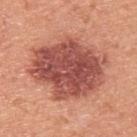Background: Automated tile analysis of the lesion measured a footprint of about 44 mm², an eccentricity of roughly 0.65, and a symmetry-axis asymmetry near 0.15. The analysis additionally found an average lesion color of about L≈51 a*≈29 b*≈28 (CIELAB), roughly 15 lightness units darker than nearby skin, and a normalized lesion–skin contrast near 10.5. The software also gave a within-lesion color-variation index near 5.5/10. The analysis additionally found a classifier nevus-likeness of about 80/100. Imaged with white-light lighting. About 9 mm across. Located on the upper back. A close-up tile cropped from a whole-body skin photograph, about 15 mm across. A male patient, approximately 45 years of age. Pathology: The biopsy diagnosis was an atypical melanocytic neoplasm — a borderline lesion of uncertain malignant potential.